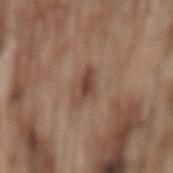Recorded during total-body skin imaging; not selected for excision or biopsy.
This image is a 15 mm lesion crop taken from a total-body photograph.
The patient is a male about 75 years old.
From the mid back.
Imaged with white-light lighting.